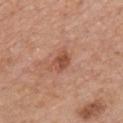Q: Is there a histopathology result?
A: no biopsy performed (imaged during a skin exam)
Q: Illumination type?
A: white-light illumination
Q: Where on the body is the lesion?
A: the chest
Q: What kind of image is this?
A: 15 mm crop, total-body photography
Q: Lesion size?
A: about 3 mm
Q: Patient demographics?
A: male, aged around 60